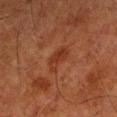No biopsy was performed on this lesion — it was imaged during a full skin examination and was not determined to be concerning.
A 15 mm crop from a total-body photograph taken for skin-cancer surveillance.
About 3.5 mm across.
The lesion is on the left lower leg.
The subject is a male aged approximately 80.
The tile uses cross-polarized illumination.
Automated image analysis of the tile measured a mean CIELAB color near L≈28 a*≈22 b*≈27, roughly 6 lightness units darker than nearby skin, and a lesion-to-skin contrast of about 6 (normalized; higher = more distinct). The analysis additionally found a classifier nevus-likeness of about 15/100 and a detector confidence of about 100 out of 100 that the crop contains a lesion.